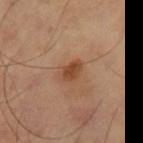Impression:
Captured during whole-body skin photography for melanoma surveillance; the lesion was not biopsied.
Clinical summary:
This is a cross-polarized tile. Cropped from a whole-body photographic skin survey; the tile spans about 15 mm. The lesion's longest dimension is about 2.5 mm. From the right thigh.